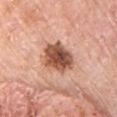notes: total-body-photography surveillance lesion; no biopsy
acquisition: 15 mm crop, total-body photography
body site: the chest
subject: male, about 80 years old
image-analysis metrics: a lesion area of about 12 mm², an eccentricity of roughly 0.6, and two-axis asymmetry of about 0.25; an average lesion color of about L≈53 a*≈24 b*≈31 (CIELAB), about 18 CIELAB-L* units darker than the surrounding skin, and a lesion-to-skin contrast of about 11.5 (normalized; higher = more distinct); a nevus-likeness score of about 35/100 and lesion-presence confidence of about 100/100
lesion size: ≈4.5 mm
lighting: white-light